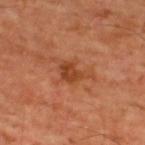site: the back
image-analysis metrics: a shape-asymmetry score of about 0.4 (0 = symmetric); a mean CIELAB color near L≈43 a*≈27 b*≈36, about 9 CIELAB-L* units darker than the surrounding skin, and a normalized lesion–skin contrast near 7; border irregularity of about 5 on a 0–10 scale
illumination: cross-polarized illumination
patient: male, aged 58 to 62
acquisition: ~15 mm tile from a whole-body skin photo